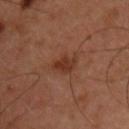patient:
  sex: male
  age_approx: 45
lighting: cross-polarized
image:
  source: total-body photography crop
  field_of_view_mm: 15
automated_metrics:
  area_mm2_approx: 4.5
  eccentricity: 0.7
  shape_asymmetry: 0.3
  nevus_likeness_0_100: 60
  lesion_detection_confidence_0_100: 100
site: chest